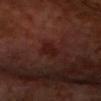biopsy_status: not biopsied; imaged during a skin examination
patient:
  sex: male
  age_approx: 70
image:
  source: total-body photography crop
  field_of_view_mm: 15
site: head or neck